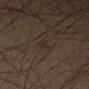{"biopsy_status": "not biopsied; imaged during a skin examination", "automated_metrics": {"cielab_L": 20, "cielab_a": 9, "cielab_b": 17, "vs_skin_darker_L": 3.0, "vs_skin_contrast_norm": 4.5, "color_variation_0_10": 0.5}, "patient": {"sex": "male", "age_approx": 70}, "site": "left thigh", "lesion_size": {"long_diameter_mm_approx": 2.5}, "lighting": "cross-polarized", "image": {"source": "total-body photography crop", "field_of_view_mm": 15}}